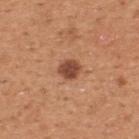{
  "biopsy_status": "not biopsied; imaged during a skin examination",
  "lighting": "white-light",
  "site": "upper back",
  "patient": {
    "sex": "male",
    "age_approx": 55
  },
  "image": {
    "source": "total-body photography crop",
    "field_of_view_mm": 15
  },
  "automated_metrics": {
    "border_irregularity_0_10": 2.0,
    "peripheral_color_asymmetry": 0.5,
    "nevus_likeness_0_100": 80
  },
  "lesion_size": {
    "long_diameter_mm_approx": 2.5
  }
}